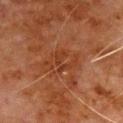| feature | finding |
|---|---|
| follow-up | total-body-photography surveillance lesion; no biopsy |
| acquisition | total-body-photography crop, ~15 mm field of view |
| lesion size | about 4 mm |
| tile lighting | cross-polarized illumination |
| automated metrics | an eccentricity of roughly 0.7 and a shape-asymmetry score of about 0.4 (0 = symmetric); an automated nevus-likeness rating near 0 out of 100 |
| patient | male, aged 78 to 82 |
| location | the front of the torso |